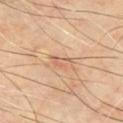workup — imaged on a skin check; not biopsied | image source — total-body-photography crop, ~15 mm field of view | subject — male, aged 63 to 67 | anatomic site — the chest.